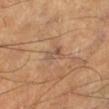Assessment:
Imaged during a routine full-body skin examination; the lesion was not biopsied and no histopathology is available.
Clinical summary:
The tile uses cross-polarized illumination. Automated tile analysis of the lesion measured an eccentricity of roughly 0.8. A male subject, aged around 60. From the leg. A 15 mm close-up tile from a total-body photography series done for melanoma screening.